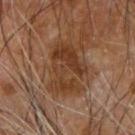Case summary:
- follow-up: catalogued during a skin exam; not biopsied
- lesion size: ≈5.5 mm
- imaging modality: ~15 mm crop, total-body skin-cancer survey
- body site: the arm
- TBP lesion metrics: a lesion color around L≈38 a*≈21 b*≈32 in CIELAB and a normalized lesion–skin contrast near 8.5; border irregularity of about 7.5 on a 0–10 scale and internal color variation of about 4.5 on a 0–10 scale
- patient: male, about 65 years old